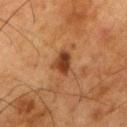This lesion was catalogued during total-body skin photography and was not selected for biopsy. The tile uses cross-polarized illumination. The total-body-photography lesion software estimated a lesion area of about 5.5 mm². The analysis additionally found a border-irregularity rating of about 2.5/10. Located on the right thigh. A 15 mm close-up extracted from a 3D total-body photography capture. The lesion's longest dimension is about 3 mm. A male subject, roughly 80 years of age.